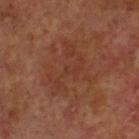Impression:
Part of a total-body skin-imaging series; this lesion was reviewed on a skin check and was not flagged for biopsy.
Context:
Automated tile analysis of the lesion measured a footprint of about 12 mm², an eccentricity of roughly 0.7, and a symmetry-axis asymmetry near 0.75. And it measured a classifier nevus-likeness of about 0/100. A male subject approximately 60 years of age. This is a cross-polarized tile. Cropped from a whole-body photographic skin survey; the tile spans about 15 mm. Located on the head or neck. About 5.5 mm across.